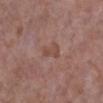Clinical impression: The lesion was photographed on a routine skin check and not biopsied; there is no pathology result. Background: A close-up tile cropped from a whole-body skin photograph, about 15 mm across. The subject is a male approximately 70 years of age. The lesion's longest dimension is about 2.5 mm. On the right lower leg. An algorithmic analysis of the crop reported border irregularity of about 3.5 on a 0–10 scale, a within-lesion color-variation index near 1/10, and a peripheral color-asymmetry measure near 0.5. And it measured lesion-presence confidence of about 100/100.